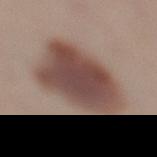This image is a 15 mm lesion crop taken from a total-body photograph. A female patient, aged around 25. On the leg.